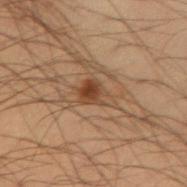notes: catalogued during a skin exam; not biopsied | automated lesion analysis: an eccentricity of roughly 0.75 and a shape-asymmetry score of about 0.35 (0 = symmetric); a mean CIELAB color near L≈36 a*≈16 b*≈27, about 9 CIELAB-L* units darker than the surrounding skin, and a lesion-to-skin contrast of about 8 (normalized; higher = more distinct) | patient: male, aged approximately 55 | size: about 4 mm | site: the left forearm | acquisition: 15 mm crop, total-body photography.